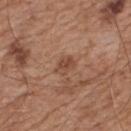* notes · catalogued during a skin exam; not biopsied